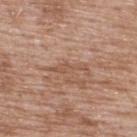{
  "biopsy_status": "not biopsied; imaged during a skin examination",
  "site": "upper back",
  "patient": {
    "sex": "male",
    "age_approx": 50
  },
  "image": {
    "source": "total-body photography crop",
    "field_of_view_mm": 15
  }
}